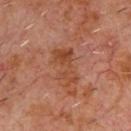Part of a total-body skin-imaging series; this lesion was reviewed on a skin check and was not flagged for biopsy.
The lesion is on the chest.
A region of skin cropped from a whole-body photographic capture, roughly 15 mm wide.
Captured under cross-polarized illumination.
Automated image analysis of the tile measured roughly 7 lightness units darker than nearby skin and a normalized lesion–skin contrast near 7. It also reported a within-lesion color-variation index near 4/10 and radial color variation of about 1.5.
The recorded lesion diameter is about 4.5 mm.
A male subject aged 58 to 62.